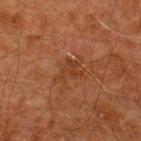Clinical impression: The lesion was tiled from a total-body skin photograph and was not biopsied. Acquisition and patient details: The subject is a male aged 58 to 62. The recorded lesion diameter is about 3 mm. On the upper back. A region of skin cropped from a whole-body photographic capture, roughly 15 mm wide. This is a cross-polarized tile.